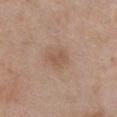Imaged during a routine full-body skin examination; the lesion was not biopsied and no histopathology is available. Automated tile analysis of the lesion measured a mean CIELAB color near L≈54 a*≈18 b*≈28 and a normalized border contrast of about 5.5. It also reported a border-irregularity rating of about 3.5/10, a within-lesion color-variation index near 1/10, and a peripheral color-asymmetry measure near 0.5. The software also gave an automated nevus-likeness rating near 5 out of 100 and lesion-presence confidence of about 100/100. A region of skin cropped from a whole-body photographic capture, roughly 15 mm wide. From the chest. Captured under white-light illumination. A female patient, approximately 60 years of age. Longest diameter approximately 2.5 mm.